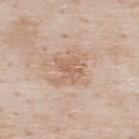{"image": {"source": "total-body photography crop", "field_of_view_mm": 15}, "site": "upper back", "patient": {"sex": "male", "age_approx": 80}, "automated_metrics": {"area_mm2_approx": 8.0, "vs_skin_darker_L": 8.0, "vs_skin_contrast_norm": 5.5}, "lighting": "white-light", "lesion_size": {"long_diameter_mm_approx": 4.0}}